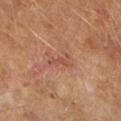Recorded during total-body skin imaging; not selected for excision or biopsy. From the arm. A 15 mm close-up tile from a total-body photography series done for melanoma screening. The subject is a female aged 58–62.